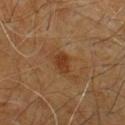Captured during whole-body skin photography for melanoma surveillance; the lesion was not biopsied.